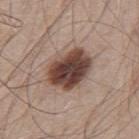Clinical impression: Part of a total-body skin-imaging series; this lesion was reviewed on a skin check and was not flagged for biopsy. Image and clinical context: On the upper back. A 15 mm close-up tile from a total-body photography series done for melanoma screening. The recorded lesion diameter is about 5 mm. Imaged with white-light lighting. A male subject roughly 50 years of age.